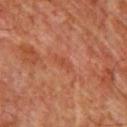| feature | finding |
|---|---|
| workup | imaged on a skin check; not biopsied |
| anatomic site | the chest |
| acquisition | 15 mm crop, total-body photography |
| subject | male, aged around 60 |